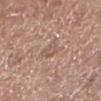Findings:
• follow-up · imaged on a skin check; not biopsied
• site · the leg
• image · ~15 mm tile from a whole-body skin photo
• patient · male, in their mid-70s